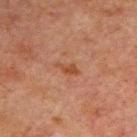– image source: 15 mm crop, total-body photography
– subject: male, aged approximately 60
– size: ≈3 mm
– body site: the left arm